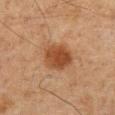Part of a total-body skin-imaging series; this lesion was reviewed on a skin check and was not flagged for biopsy.